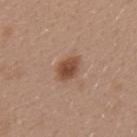This lesion was catalogued during total-body skin photography and was not selected for biopsy.
This is a white-light tile.
A lesion tile, about 15 mm wide, cut from a 3D total-body photograph.
A female subject, aged 38 to 42.
Located on the back.
The total-body-photography lesion software estimated an eccentricity of roughly 0.7 and two-axis asymmetry of about 0.15. The software also gave an automated nevus-likeness rating near 95 out of 100 and lesion-presence confidence of about 100/100.